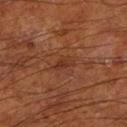Clinical impression:
This lesion was catalogued during total-body skin photography and was not selected for biopsy.
Image and clinical context:
On the left lower leg. The tile uses cross-polarized illumination. The lesion-visualizer software estimated a lesion area of about 3.5 mm², an eccentricity of roughly 0.85, and a symmetry-axis asymmetry near 0.35. It also reported a border-irregularity index near 3.5/10 and radial color variation of about 0.5. And it measured a lesion-detection confidence of about 100/100. This image is a 15 mm lesion crop taken from a total-body photograph. The patient is a male about 65 years old. Longest diameter approximately 3 mm.